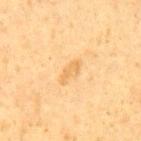No biopsy was performed on this lesion — it was imaged during a full skin examination and was not determined to be concerning.